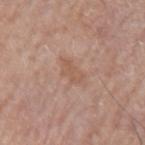Impression:
This lesion was catalogued during total-body skin photography and was not selected for biopsy.
Acquisition and patient details:
The lesion is on the left upper arm. The lesion's longest dimension is about 3 mm. A 15 mm close-up extracted from a 3D total-body photography capture. Automated tile analysis of the lesion measured a within-lesion color-variation index near 1.5/10 and peripheral color asymmetry of about 0.5. The patient is a male in their mid- to late 70s.